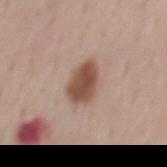- biopsy status — total-body-photography surveillance lesion; no biopsy
- body site — the mid back
- imaging modality — ~15 mm crop, total-body skin-cancer survey
- automated metrics — a footprint of about 10 mm², an eccentricity of roughly 0.65, and two-axis asymmetry of about 0.2; an automated nevus-likeness rating near 100 out of 100
- patient — male, aged 53 to 57